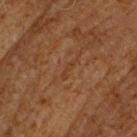biopsy status — imaged on a skin check; not biopsied | image source — 15 mm crop, total-body photography | site — the head or neck | TBP lesion metrics — a mean CIELAB color near L≈31 a*≈17 b*≈28, roughly 4 lightness units darker than nearby skin, and a lesion-to-skin contrast of about 4.5 (normalized; higher = more distinct) | subject — male, roughly 65 years of age.